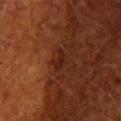Clinical impression: No biopsy was performed on this lesion — it was imaged during a full skin examination and was not determined to be concerning. Clinical summary: A close-up tile cropped from a whole-body skin photograph, about 15 mm across. The lesion is on the upper back. Approximately 3 mm at its widest. The subject is a female aged 48 to 52.